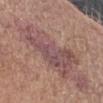Impression: The lesion was photographed on a routine skin check and not biopsied; there is no pathology result. Image and clinical context: On the left forearm. The recorded lesion diameter is about 8 mm. A close-up tile cropped from a whole-body skin photograph, about 15 mm across. The patient is a female aged approximately 65.